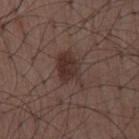workup: imaged on a skin check; not biopsied | acquisition: ~15 mm crop, total-body skin-cancer survey | subject: male, about 50 years old | location: the back.